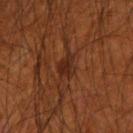Notes:
• notes — catalogued during a skin exam; not biopsied
• site — the right upper arm
• tile lighting — cross-polarized illumination
• lesion size — ≈3.5 mm
• image-analysis metrics — a classifier nevus-likeness of about 85/100
• image — ~15 mm tile from a whole-body skin photo
• subject — male, aged 68 to 72